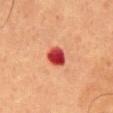Case summary:
– follow-up — catalogued during a skin exam; not biopsied
– patient — male, about 55 years old
– image — total-body-photography crop, ~15 mm field of view
– anatomic site — the abdomen